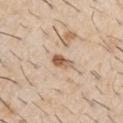A male patient, in their 60s.
The lesion is located on the right upper arm.
Measured at roughly 3 mm in maximum diameter.
A 15 mm crop from a total-body photograph taken for skin-cancer surveillance.
This is a white-light tile.